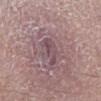Impression: The lesion was tiled from a total-body skin photograph and was not biopsied. Acquisition and patient details: Imaged with white-light lighting. The recorded lesion diameter is about 3.5 mm. On the right lower leg. The total-body-photography lesion software estimated a lesion-detection confidence of about 50/100. A male patient about 55 years old. This image is a 15 mm lesion crop taken from a total-body photograph.